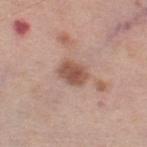site: the right thigh; illumination: white-light illumination; subject: female, in their mid-60s; image source: ~15 mm tile from a whole-body skin photo; size: ≈3 mm.